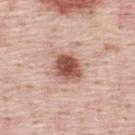{"biopsy_status": "not biopsied; imaged during a skin examination", "automated_metrics": {"cielab_L": 54, "cielab_a": 23, "cielab_b": 27, "vs_skin_darker_L": 16.0, "vs_skin_contrast_norm": 10.5, "border_irregularity_0_10": 2.0, "color_variation_0_10": 4.5, "peripheral_color_asymmetry": 1.5, "nevus_likeness_0_100": 95, "lesion_detection_confidence_0_100": 100}, "patient": {"sex": "male", "age_approx": 45}, "image": {"source": "total-body photography crop", "field_of_view_mm": 15}, "site": "back", "lesion_size": {"long_diameter_mm_approx": 3.5}, "lighting": "white-light"}